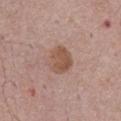Recorded during total-body skin imaging; not selected for excision or biopsy.